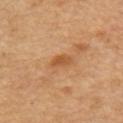Image and clinical context: Cropped from a total-body skin-imaging series; the visible field is about 15 mm. The patient is a male approximately 55 years of age. This is a cross-polarized tile. Located on the right upper arm. About 3 mm across. Automated tile analysis of the lesion measured border irregularity of about 2 on a 0–10 scale, a within-lesion color-variation index near 3/10, and radial color variation of about 1.